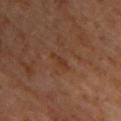Captured during whole-body skin photography for melanoma surveillance; the lesion was not biopsied. The patient is a male approximately 60 years of age. A 15 mm close-up extracted from a 3D total-body photography capture. From the upper back. Automated tile analysis of the lesion measured an eccentricity of roughly 0.95 and a shape-asymmetry score of about 0.5 (0 = symmetric).